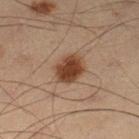Imaged during a routine full-body skin examination; the lesion was not biopsied and no histopathology is available.
The total-body-photography lesion software estimated a mean CIELAB color near L≈34 a*≈17 b*≈26 and about 12 CIELAB-L* units darker than the surrounding skin.
A region of skin cropped from a whole-body photographic capture, roughly 15 mm wide.
The lesion is located on the leg.
The lesion's longest dimension is about 4 mm.
A male subject approximately 55 years of age.